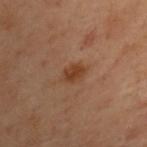Q: Was a biopsy performed?
A: no biopsy performed (imaged during a skin exam)
Q: Lesion location?
A: the upper back
Q: What are the patient's age and sex?
A: female, aged 58–62
Q: What kind of image is this?
A: ~15 mm tile from a whole-body skin photo
Q: Automated lesion metrics?
A: a footprint of about 4.5 mm², an eccentricity of roughly 0.7, and two-axis asymmetry of about 0.2; a lesion color around L≈35 a*≈19 b*≈29 in CIELAB, about 7 CIELAB-L* units darker than the surrounding skin, and a lesion-to-skin contrast of about 7.5 (normalized; higher = more distinct); a classifier nevus-likeness of about 90/100 and a detector confidence of about 100 out of 100 that the crop contains a lesion
Q: Illumination type?
A: cross-polarized illumination
Q: How large is the lesion?
A: about 3 mm